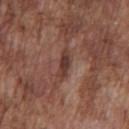Recorded during total-body skin imaging; not selected for excision or biopsy. Approximately 3.5 mm at its widest. Located on the chest. A male patient roughly 75 years of age. Automated tile analysis of the lesion measured an area of roughly 4.5 mm², an eccentricity of roughly 0.9, and a shape-asymmetry score of about 0.2 (0 = symmetric). The software also gave an average lesion color of about L≈38 a*≈20 b*≈23 (CIELAB) and about 9 CIELAB-L* units darker than the surrounding skin. And it measured a border-irregularity rating of about 2.5/10. The analysis additionally found lesion-presence confidence of about 90/100. Imaged with white-light lighting. A 15 mm close-up tile from a total-body photography series done for melanoma screening.